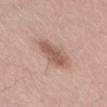Acquisition and patient details:
This is a white-light tile. Located on the left thigh. The recorded lesion diameter is about 5 mm. A 15 mm crop from a total-body photograph taken for skin-cancer surveillance. A male subject, approximately 60 years of age.